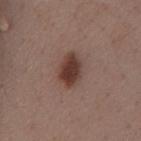Findings:
• biopsy status · total-body-photography surveillance lesion; no biopsy
• body site · the chest
• illumination · white-light illumination
• patient · female, about 55 years old
• image source · ~15 mm tile from a whole-body skin photo